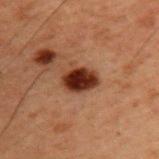Assessment: Recorded during total-body skin imaging; not selected for excision or biopsy. Context: A region of skin cropped from a whole-body photographic capture, roughly 15 mm wide. On the upper back. A male patient, aged around 60.